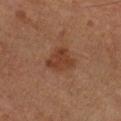<tbp_lesion>
  <biopsy_status>not biopsied; imaged during a skin examination</biopsy_status>
  <lesion_size>
    <long_diameter_mm_approx>3.5</long_diameter_mm_approx>
  </lesion_size>
  <image>
    <source>total-body photography crop</source>
    <field_of_view_mm>15</field_of_view_mm>
  </image>
  <lighting>cross-polarized</lighting>
  <automated_metrics>
    <cielab_L>38</cielab_L>
    <cielab_a>22</cielab_a>
    <cielab_b>30</cielab_b>
    <vs_skin_darker_L>8.0</vs_skin_darker_L>
    <border_irregularity_0_10>3.5</border_irregularity_0_10>
    <color_variation_0_10>2.5</color_variation_0_10>
  </automated_metrics>
  <site>left forearm</site>
  <patient>
    <sex>male</sex>
    <age_approx>60</age_approx>
  </patient>
</tbp_lesion>